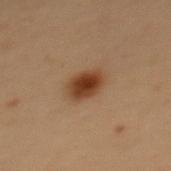Assessment:
Captured during whole-body skin photography for melanoma surveillance; the lesion was not biopsied.
Image and clinical context:
The recorded lesion diameter is about 3 mm. A female patient, aged 48 to 52. The tile uses cross-polarized illumination. Located on the back. Cropped from a total-body skin-imaging series; the visible field is about 15 mm.